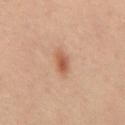{
  "biopsy_status": "not biopsied; imaged during a skin examination"
}